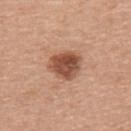This lesion was catalogued during total-body skin photography and was not selected for biopsy. From the upper back. A male patient, aged 53–57. Cropped from a total-body skin-imaging series; the visible field is about 15 mm.